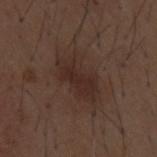biopsy status: imaged on a skin check; not biopsied | location: the mid back | subject: male, aged around 50 | size: ≈6 mm | tile lighting: white-light illumination | imaging modality: ~15 mm tile from a whole-body skin photo | TBP lesion metrics: a lesion area of about 13 mm² and a shape-asymmetry score of about 0.2 (0 = symmetric); border irregularity of about 3.5 on a 0–10 scale and radial color variation of about 1.